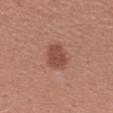Clinical impression: The lesion was tiled from a total-body skin photograph and was not biopsied. Clinical summary: A 15 mm close-up extracted from a 3D total-body photography capture. This is a white-light tile. On the left forearm. A female patient roughly 45 years of age.